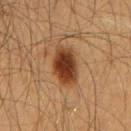Captured during whole-body skin photography for melanoma surveillance; the lesion was not biopsied. This is a cross-polarized tile. A 15 mm close-up tile from a total-body photography series done for melanoma screening. A male patient, aged 58–62. From the front of the torso.